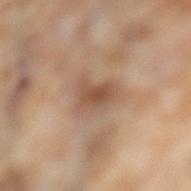workup = imaged on a skin check; not biopsied
tile lighting = cross-polarized illumination
subject = female, roughly 55 years of age
lesion size = about 4 mm
acquisition = 15 mm crop, total-body photography
location = the leg